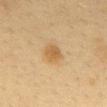Q: Was this lesion biopsied?
A: no biopsy performed (imaged during a skin exam)
Q: What are the patient's age and sex?
A: female, aged approximately 50
Q: How was this image acquired?
A: total-body-photography crop, ~15 mm field of view
Q: Where on the body is the lesion?
A: the mid back